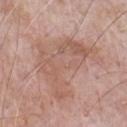Recorded during total-body skin imaging; not selected for excision or biopsy.
The patient is a male approximately 70 years of age.
A region of skin cropped from a whole-body photographic capture, roughly 15 mm wide.
From the chest.
Approximately 8 mm at its widest.
This is a white-light tile.